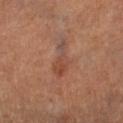Imaged during a routine full-body skin examination; the lesion was not biopsied and no histopathology is available.
Imaged with cross-polarized lighting.
A male patient, about 85 years old or older.
The lesion-visualizer software estimated an area of roughly 6.5 mm², an eccentricity of roughly 0.95, and two-axis asymmetry of about 0.45. The analysis additionally found a lesion color around L≈45 a*≈21 b*≈28 in CIELAB and a lesion–skin lightness drop of about 7.
On the left thigh.
A region of skin cropped from a whole-body photographic capture, roughly 15 mm wide.
The lesion's longest dimension is about 5.5 mm.